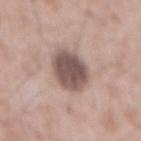Clinical impression:
The lesion was photographed on a routine skin check and not biopsied; there is no pathology result.
Background:
The tile uses white-light illumination. A region of skin cropped from a whole-body photographic capture, roughly 15 mm wide. Approximately 5.5 mm at its widest. The subject is a male roughly 50 years of age. On the mid back.